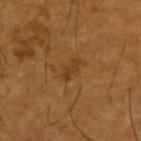<lesion>
  <biopsy_status>not biopsied; imaged during a skin examination</biopsy_status>
  <patient>
    <sex>male</sex>
    <age_approx>65</age_approx>
  </patient>
  <image>
    <source>total-body photography crop</source>
    <field_of_view_mm>15</field_of_view_mm>
  </image>
  <site>left upper arm</site>
</lesion>